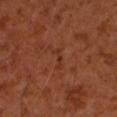Assessment:
The lesion was photographed on a routine skin check and not biopsied; there is no pathology result.
Background:
A male patient aged 58–62. A close-up tile cropped from a whole-body skin photograph, about 15 mm across. The lesion is on the left forearm.